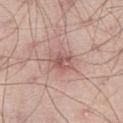<case>
  <biopsy_status>not biopsied; imaged during a skin examination</biopsy_status>
  <image>
    <source>total-body photography crop</source>
    <field_of_view_mm>15</field_of_view_mm>
  </image>
  <site>right thigh</site>
  <lighting>white-light</lighting>
  <lesion_size>
    <long_diameter_mm_approx>3.5</long_diameter_mm_approx>
  </lesion_size>
  <patient>
    <sex>male</sex>
    <age_approx>65</age_approx>
  </patient>
</case>